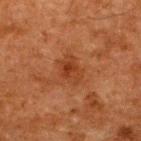Captured under cross-polarized illumination.
A 15 mm close-up tile from a total-body photography series done for melanoma screening.
The lesion is on the upper back.
Approximately 3.5 mm at its widest.
A male patient in their 60s.